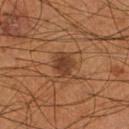Clinical impression: No biopsy was performed on this lesion — it was imaged during a full skin examination and was not determined to be concerning. Context: Captured under cross-polarized illumination. The lesion is on the right lower leg. A 15 mm close-up tile from a total-body photography series done for melanoma screening. The lesion's longest dimension is about 3.5 mm. A male patient in their mid-50s. Automated image analysis of the tile measured an outline eccentricity of about 0.65 (0 = round, 1 = elongated) and a symmetry-axis asymmetry near 0.25. The software also gave a normalized border contrast of about 8. It also reported a nevus-likeness score of about 70/100 and a lesion-detection confidence of about 100/100.